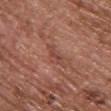follow-up = catalogued during a skin exam; not biopsied
subject = female, in their mid- to late 60s
body site = the chest
acquisition = ~15 mm tile from a whole-body skin photo